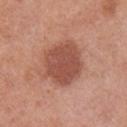The lesion was tiled from a total-body skin photograph and was not biopsied. Measured at roughly 5.5 mm in maximum diameter. Located on the chest. A 15 mm close-up extracted from a 3D total-body photography capture. This is a white-light tile. The subject is a male aged 68 to 72. Automated tile analysis of the lesion measured a lesion–skin lightness drop of about 12. The analysis additionally found a lesion-detection confidence of about 100/100.